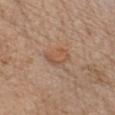{"biopsy_status": "not biopsied; imaged during a skin examination", "lesion_size": {"long_diameter_mm_approx": 3.5}, "lighting": "white-light", "patient": {"sex": "female", "age_approx": 70}, "image": {"source": "total-body photography crop", "field_of_view_mm": 15}, "site": "chest"}